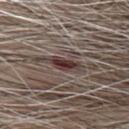No biopsy was performed on this lesion — it was imaged during a full skin examination and was not determined to be concerning. The lesion's longest dimension is about 3.5 mm. A 15 mm close-up tile from a total-body photography series done for melanoma screening. On the chest. A male subject, aged approximately 80.